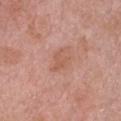No biopsy was performed on this lesion — it was imaged during a full skin examination and was not determined to be concerning.
The subject is a female aged 63 to 67.
A 15 mm close-up extracted from a 3D total-body photography capture.
The lesion-visualizer software estimated a within-lesion color-variation index near 1.5/10. The software also gave an automated nevus-likeness rating near 0 out of 100 and a lesion-detection confidence of about 100/100.
Approximately 3 mm at its widest.
From the chest.